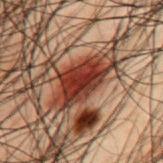  biopsy_status: not biopsied; imaged during a skin examination
  site: mid back
  lesion_size:
    long_diameter_mm_approx: 7.0
  automated_metrics:
    area_mm2_approx: 17.0
    shape_asymmetry: 0.25
    cielab_L: 27
    cielab_a: 22
    cielab_b: 24
    vs_skin_darker_L: 14.0
    vs_skin_contrast_norm: 13.0
    border_irregularity_0_10: 4.0
    color_variation_0_10: 6.0
    peripheral_color_asymmetry: 2.0
  patient:
    sex: male
    age_approx: 50
  lighting: cross-polarized
  image:
    source: total-body photography crop
    field_of_view_mm: 15